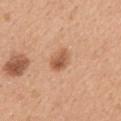Assessment: Part of a total-body skin-imaging series; this lesion was reviewed on a skin check and was not flagged for biopsy. Clinical summary: From the back. About 3 mm across. A lesion tile, about 15 mm wide, cut from a 3D total-body photograph. Imaged with white-light lighting. The subject is a female aged approximately 30.